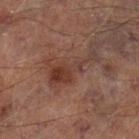A 15 mm crop from a total-body photograph taken for skin-cancer surveillance.
Automated image analysis of the tile measured a border-irregularity rating of about 9.5/10, a within-lesion color-variation index near 3/10, and a peripheral color-asymmetry measure near 1. And it measured a nevus-likeness score of about 15/100.
Measured at roughly 6 mm in maximum diameter.
From the left lower leg.
A male subject, aged approximately 65.